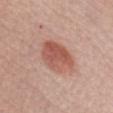Q: Who is the patient?
A: female, roughly 50 years of age
Q: Lesion location?
A: the chest
Q: How was the tile lit?
A: white-light illumination
Q: How large is the lesion?
A: ~5 mm (longest diameter)
Q: What kind of image is this?
A: total-body-photography crop, ~15 mm field of view